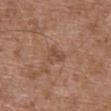Q: Is there a histopathology result?
A: imaged on a skin check; not biopsied
Q: What are the patient's age and sex?
A: male, aged 73 to 77
Q: What lighting was used for the tile?
A: white-light
Q: What did automated image analysis measure?
A: an eccentricity of roughly 0.8; an average lesion color of about L≈48 a*≈20 b*≈29 (CIELAB), roughly 7 lightness units darker than nearby skin, and a lesion-to-skin contrast of about 5.5 (normalized; higher = more distinct); a nevus-likeness score of about 0/100
Q: What kind of image is this?
A: 15 mm crop, total-body photography
Q: Lesion size?
A: about 2.5 mm
Q: Lesion location?
A: the abdomen